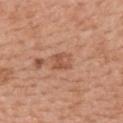Q: Is there a histopathology result?
A: no biopsy performed (imaged during a skin exam)
Q: Lesion location?
A: the upper back
Q: What is the lesion's diameter?
A: ~2.5 mm (longest diameter)
Q: Patient demographics?
A: female, aged 33 to 37
Q: Illumination type?
A: white-light illumination
Q: What kind of image is this?
A: total-body-photography crop, ~15 mm field of view